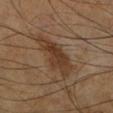notes: catalogued during a skin exam; not biopsied | lesion size: ≈6.5 mm | illumination: cross-polarized illumination | image: ~15 mm crop, total-body skin-cancer survey | site: the leg | subject: male, aged around 65.